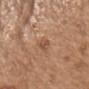<tbp_lesion>
<image>
  <source>total-body photography crop</source>
  <field_of_view_mm>15</field_of_view_mm>
</image>
<automated_metrics>
  <vs_skin_darker_L>7.0</vs_skin_darker_L>
  <vs_skin_contrast_norm>5.5</vs_skin_contrast_norm>
  <border_irregularity_0_10>2.5</border_irregularity_0_10>
  <color_variation_0_10>2.0</color_variation_0_10>
  <peripheral_color_asymmetry>0.5</peripheral_color_asymmetry>
  <nevus_likeness_0_100>0</nevus_likeness_0_100>
  <lesion_detection_confidence_0_100>100</lesion_detection_confidence_0_100>
</automated_metrics>
<patient>
  <sex>male</sex>
  <age_approx>70</age_approx>
</patient>
<lighting>white-light</lighting>
<lesion_size>
  <long_diameter_mm_approx>2.5</long_diameter_mm_approx>
</lesion_size>
<site>head or neck</site>
</tbp_lesion>